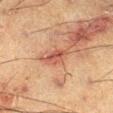The lesion was photographed on a routine skin check and not biopsied; there is no pathology result. A male patient, approximately 60 years of age. A region of skin cropped from a whole-body photographic capture, roughly 15 mm wide. From the right lower leg.